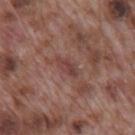Captured during whole-body skin photography for melanoma surveillance; the lesion was not biopsied. The lesion is on the mid back. A lesion tile, about 15 mm wide, cut from a 3D total-body photograph. The lesion's longest dimension is about 3 mm. A male subject, about 70 years old.